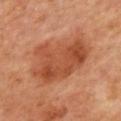Q: Is there a histopathology result?
A: catalogued during a skin exam; not biopsied